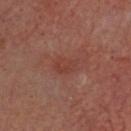Context:
Located on the head or neck. The subject is a male aged around 65. A 15 mm close-up tile from a total-body photography series done for melanoma screening.